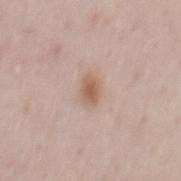Recorded during total-body skin imaging; not selected for excision or biopsy. Cropped from a whole-body photographic skin survey; the tile spans about 15 mm. The lesion's longest dimension is about 2.5 mm. Captured under white-light illumination. From the back. The subject is a female approximately 50 years of age. The lesion-visualizer software estimated an area of roughly 4 mm², an eccentricity of roughly 0.8, and two-axis asymmetry of about 0.15. The software also gave a lesion color around L≈59 a*≈19 b*≈28 in CIELAB and a lesion-to-skin contrast of about 8 (normalized; higher = more distinct). It also reported a within-lesion color-variation index near 2.5/10 and a peripheral color-asymmetry measure near 1.